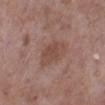imaging modality: total-body-photography crop, ~15 mm field of view
image-analysis metrics: border irregularity of about 2 on a 0–10 scale, a color-variation rating of about 2.5/10, and radial color variation of about 1
patient: male, aged 68–72
anatomic site: the left lower leg
diameter: about 4.5 mm